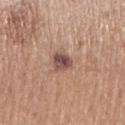<case>
<biopsy_status>not biopsied; imaged during a skin examination</biopsy_status>
<lighting>white-light</lighting>
<lesion_size>
  <long_diameter_mm_approx>2.5</long_diameter_mm_approx>
</lesion_size>
<image>
  <source>total-body photography crop</source>
  <field_of_view_mm>15</field_of_view_mm>
</image>
<site>right upper arm</site>
<patient>
  <sex>female</sex>
  <age_approx>65</age_approx>
</patient>
</case>